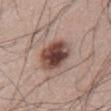Assessment: Imaged during a routine full-body skin examination; the lesion was not biopsied and no histopathology is available. Acquisition and patient details: On the abdomen. The recorded lesion diameter is about 5 mm. This image is a 15 mm lesion crop taken from a total-body photograph. This is a white-light tile. The subject is a male in their mid- to late 60s.